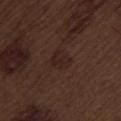The lesion was photographed on a routine skin check and not biopsied; there is no pathology result.
A male patient, about 70 years old.
Measured at roughly 3 mm in maximum diameter.
The tile uses white-light illumination.
Located on the back.
A 15 mm close-up extracted from a 3D total-body photography capture.